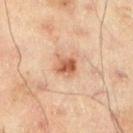lighting = cross-polarized illumination
acquisition = total-body-photography crop, ~15 mm field of view
lesion size = ~2.5 mm (longest diameter)
patient = male, in their mid- to late 40s
anatomic site = the left lower leg
automated metrics = an area of roughly 4.5 mm² and two-axis asymmetry of about 0.2; border irregularity of about 2 on a 0–10 scale, internal color variation of about 3 on a 0–10 scale, and peripheral color asymmetry of about 1; a classifier nevus-likeness of about 80/100 and a lesion-detection confidence of about 100/100